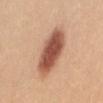Impression:
No biopsy was performed on this lesion — it was imaged during a full skin examination and was not determined to be concerning.
Clinical summary:
The lesion is located on the back. Approximately 7 mm at its widest. Captured under white-light illumination. A female subject aged 23 to 27. This image is a 15 mm lesion crop taken from a total-body photograph.